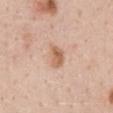Imaged during a routine full-body skin examination; the lesion was not biopsied and no histopathology is available. Cropped from a whole-body photographic skin survey; the tile spans about 15 mm. The tile uses white-light illumination. The lesion is located on the abdomen. The lesion's longest dimension is about 3 mm. A female subject, aged 43–47. Automated tile analysis of the lesion measured an area of roughly 5 mm² and a shape eccentricity near 0.75. It also reported an average lesion color of about L≈62 a*≈20 b*≈32 (CIELAB) and a normalized lesion–skin contrast near 7.5.